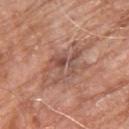biopsy status: catalogued during a skin exam; not biopsied | location: the upper back | lesion size: ~5 mm (longest diameter) | image: ~15 mm tile from a whole-body skin photo | automated metrics: an average lesion color of about L≈51 a*≈22 b*≈26 (CIELAB), about 9 CIELAB-L* units darker than the surrounding skin, and a lesion-to-skin contrast of about 6.5 (normalized; higher = more distinct) | patient: male, approximately 80 years of age | tile lighting: white-light.